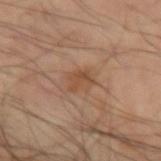This lesion was catalogued during total-body skin photography and was not selected for biopsy.
The lesion is on the left forearm.
Measured at roughly 3 mm in maximum diameter.
A male subject, approximately 65 years of age.
A lesion tile, about 15 mm wide, cut from a 3D total-body photograph.
Imaged with cross-polarized lighting.
The total-body-photography lesion software estimated a lesion area of about 4 mm². And it measured a border-irregularity rating of about 3.5/10, internal color variation of about 2.5 on a 0–10 scale, and peripheral color asymmetry of about 1.